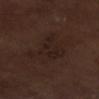Assessment:
Captured during whole-body skin photography for melanoma surveillance; the lesion was not biopsied.
Image and clinical context:
This is a white-light tile. A male patient, aged around 70. The lesion-visualizer software estimated a footprint of about 18 mm², a shape eccentricity near 0.8, and a shape-asymmetry score of about 0.4 (0 = symmetric). It also reported border irregularity of about 6 on a 0–10 scale, a within-lesion color-variation index near 2.5/10, and a peripheral color-asymmetry measure near 1. Approximately 6.5 mm at its widest. This image is a 15 mm lesion crop taken from a total-body photograph. Located on the right lower leg.